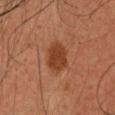• biopsy status: no biopsy performed (imaged during a skin exam)
• image source: total-body-photography crop, ~15 mm field of view
• lesion size: ≈3.5 mm
• subject: male, about 35 years old
• anatomic site: the head or neck